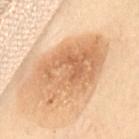Recorded during total-body skin imaging; not selected for excision or biopsy.
Cropped from a whole-body photographic skin survey; the tile spans about 15 mm.
Located on the abdomen.
Imaged with cross-polarized lighting.
A female patient, about 60 years old.
An algorithmic analysis of the crop reported border irregularity of about 2.5 on a 0–10 scale and a color-variation rating of about 5.5/10.
Longest diameter approximately 9 mm.